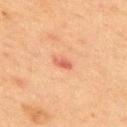biopsy status: total-body-photography surveillance lesion; no biopsy
tile lighting: cross-polarized
body site: the upper back
acquisition: total-body-photography crop, ~15 mm field of view
patient: male, in their 70s
diameter: ≈2.5 mm